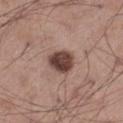The lesion was photographed on a routine skin check and not biopsied; there is no pathology result. Captured under white-light illumination. Automated image analysis of the tile measured a shape eccentricity near 0.55 and two-axis asymmetry of about 0.2. The software also gave an average lesion color of about L≈42 a*≈19 b*≈22 (CIELAB), a lesion–skin lightness drop of about 17, and a normalized lesion–skin contrast near 12.5. It also reported a border-irregularity rating of about 2/10 and internal color variation of about 4 on a 0–10 scale. It also reported an automated nevus-likeness rating near 45 out of 100 and lesion-presence confidence of about 100/100. A roughly 15 mm field-of-view crop from a total-body skin photograph. Longest diameter approximately 3.5 mm. A male subject, in their mid- to late 50s. The lesion is on the left thigh.